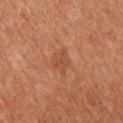<lesion>
<biopsy_status>not biopsied; imaged during a skin examination</biopsy_status>
<patient>
  <sex>male</sex>
  <age_approx>55</age_approx>
</patient>
<site>right upper arm</site>
<lesion_size>
  <long_diameter_mm_approx>3.0</long_diameter_mm_approx>
</lesion_size>
<lighting>white-light</lighting>
<image>
  <source>total-body photography crop</source>
  <field_of_view_mm>15</field_of_view_mm>
</image>
<automated_metrics>
  <cielab_L>51</cielab_L>
  <cielab_a>26</cielab_a>
  <cielab_b>36</cielab_b>
  <vs_skin_darker_L>7.0</vs_skin_darker_L>
</automated_metrics>
</lesion>